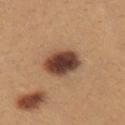Context:
The subject is a female about 25 years old. Located on the chest. A 15 mm close-up tile from a total-body photography series done for melanoma screening.